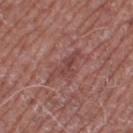notes: no biopsy performed (imaged during a skin exam).